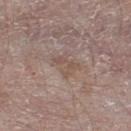{
  "biopsy_status": "not biopsied; imaged during a skin examination",
  "automated_metrics": {
    "cielab_L": 52,
    "cielab_a": 15,
    "cielab_b": 23,
    "vs_skin_darker_L": 6.0,
    "vs_skin_contrast_norm": 5.0,
    "border_irregularity_0_10": 4.0,
    "color_variation_0_10": 2.5,
    "peripheral_color_asymmetry": 1.0,
    "nevus_likeness_0_100": 0
  },
  "site": "right lower leg",
  "patient": {
    "sex": "male",
    "age_approx": 65
  },
  "image": {
    "source": "total-body photography crop",
    "field_of_view_mm": 15
  }
}